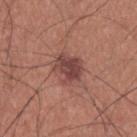Background: From the upper back. Automated image analysis of the tile measured an area of roughly 9 mm² and a symmetry-axis asymmetry near 0.25. The software also gave a border-irregularity rating of about 2.5/10, a color-variation rating of about 4/10, and a peripheral color-asymmetry measure near 1.5. A male patient, aged around 35. A 15 mm close-up extracted from a 3D total-body photography capture. This is a white-light tile.